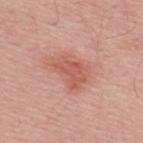No biopsy was performed on this lesion — it was imaged during a full skin examination and was not determined to be concerning.
A lesion tile, about 15 mm wide, cut from a 3D total-body photograph.
The patient is a male approximately 30 years of age.
The tile uses white-light illumination.
From the upper back.
Measured at roughly 4.5 mm in maximum diameter.